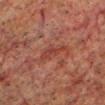No biopsy was performed on this lesion — it was imaged during a full skin examination and was not determined to be concerning. The lesion's longest dimension is about 4 mm. A male patient, in their 60s. The tile uses cross-polarized illumination. The lesion-visualizer software estimated a lesion color around L≈31 a*≈23 b*≈23 in CIELAB, a lesion–skin lightness drop of about 5, and a normalized border contrast of about 5.5. The software also gave a border-irregularity rating of about 5.5/10, a color-variation rating of about 1.5/10, and a peripheral color-asymmetry measure near 0.5. And it measured a nevus-likeness score of about 0/100. From the head or neck. Cropped from a total-body skin-imaging series; the visible field is about 15 mm.